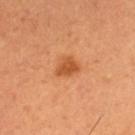Clinical impression:
The lesion was tiled from a total-body skin photograph and was not biopsied.
Acquisition and patient details:
A male subject aged 48 to 52. Approximately 3 mm at its widest. This image is a 15 mm lesion crop taken from a total-body photograph. From the left thigh.